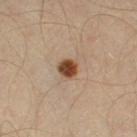- follow-up: no biopsy performed (imaged during a skin exam)
- lighting: cross-polarized illumination
- site: the leg
- diameter: about 2.5 mm
- imaging modality: total-body-photography crop, ~15 mm field of view
- subject: male, approximately 40 years of age